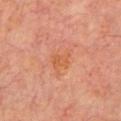automated metrics: a lesion area of about 4 mm², an outline eccentricity of about 0.7 (0 = round, 1 = elongated), and a symmetry-axis asymmetry near 0.35; a lesion color around L≈56 a*≈28 b*≈38 in CIELAB and about 5 CIELAB-L* units darker than the surrounding skin; a border-irregularity index near 3.5/10, a within-lesion color-variation index near 2/10, and peripheral color asymmetry of about 0.5; an automated nevus-likeness rating near 0 out of 100 and a detector confidence of about 100 out of 100 that the crop contains a lesion | subject: male, approximately 60 years of age | site: the front of the torso | size: about 2.5 mm | illumination: cross-polarized illumination | acquisition: total-body-photography crop, ~15 mm field of view.